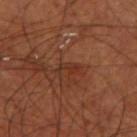Impression: Imaged during a routine full-body skin examination; the lesion was not biopsied and no histopathology is available. Acquisition and patient details: A male patient, about 70 years old. On the left thigh. A region of skin cropped from a whole-body photographic capture, roughly 15 mm wide.